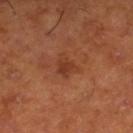  site: right lower leg
  patient:
    sex: male
    age_approx: 65
  image:
    source: total-body photography crop
    field_of_view_mm: 15